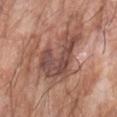A male patient, in their 60s.
From the left forearm.
The tile uses white-light illumination.
The total-body-photography lesion software estimated an automated nevus-likeness rating near 0 out of 100 and a detector confidence of about 95 out of 100 that the crop contains a lesion.
A 15 mm close-up tile from a total-body photography series done for melanoma screening.